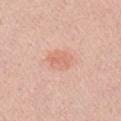Notes:
– workup: no biopsy performed (imaged during a skin exam)
– subject: male, roughly 65 years of age
– image source: ~15 mm tile from a whole-body skin photo
– site: the right upper arm